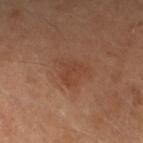No biopsy was performed on this lesion — it was imaged during a full skin examination and was not determined to be concerning.
On the right forearm.
A 15 mm crop from a total-body photograph taken for skin-cancer surveillance.
A female subject, aged around 60.